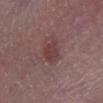Impression:
The lesion was tiled from a total-body skin photograph and was not biopsied.
Context:
A male subject aged approximately 80. A 15 mm close-up tile from a total-body photography series done for melanoma screening. The lesion-visualizer software estimated a lesion area of about 6 mm², an eccentricity of roughly 0.35, and a symmetry-axis asymmetry near 0.25. The lesion is on the right lower leg. This is a white-light tile.